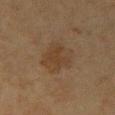Clinical impression: Imaged during a routine full-body skin examination; the lesion was not biopsied and no histopathology is available. Acquisition and patient details: Measured at roughly 4.5 mm in maximum diameter. The tile uses cross-polarized illumination. From the arm. A region of skin cropped from a whole-body photographic capture, roughly 15 mm wide. Automated image analysis of the tile measured a lesion area of about 13 mm², an eccentricity of roughly 0.65, and a symmetry-axis asymmetry near 0.2. A female subject, aged around 50.